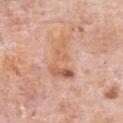Notes:
* notes — imaged on a skin check; not biopsied
* patient — male, aged around 80
* body site — the chest
* tile lighting — white-light illumination
* diameter — ~5.5 mm (longest diameter)
* image — ~15 mm tile from a whole-body skin photo